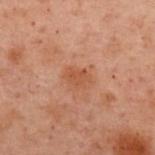The lesion was photographed on a routine skin check and not biopsied; there is no pathology result.
Captured under cross-polarized illumination.
The lesion is located on the upper back.
A female patient roughly 55 years of age.
About 2.5 mm across.
A 15 mm close-up tile from a total-body photography series done for melanoma screening.